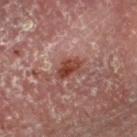Impression: The lesion was photographed on a routine skin check and not biopsied; there is no pathology result. Clinical summary: Located on the right upper arm. Cropped from a whole-body photographic skin survey; the tile spans about 15 mm. This is a cross-polarized tile. A male patient, approximately 55 years of age. The recorded lesion diameter is about 3 mm.